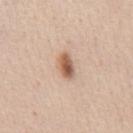No biopsy was performed on this lesion — it was imaged during a full skin examination and was not determined to be concerning.